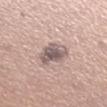The lesion was photographed on a routine skin check and not biopsied; there is no pathology result. A lesion tile, about 15 mm wide, cut from a 3D total-body photograph. From the right lower leg. An algorithmic analysis of the crop reported a lesion area of about 9 mm² and a shape-asymmetry score of about 0.25 (0 = symmetric). The software also gave lesion-presence confidence of about 100/100. About 4 mm across. A female patient, roughly 40 years of age. Imaged with white-light lighting.